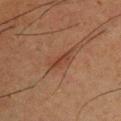| feature | finding |
|---|---|
| follow-up | imaged on a skin check; not biopsied |
| image source | total-body-photography crop, ~15 mm field of view |
| patient | male, aged approximately 35 |
| location | the front of the torso |
| lesion diameter | ~3.5 mm (longest diameter) |